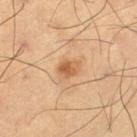Impression: The lesion was photographed on a routine skin check and not biopsied; there is no pathology result. Image and clinical context: The lesion is located on the left thigh. A male subject, roughly 65 years of age. Cropped from a total-body skin-imaging series; the visible field is about 15 mm. The tile uses cross-polarized illumination. Longest diameter approximately 3 mm.